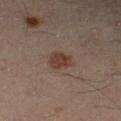Case summary:
• follow-up · catalogued during a skin exam; not biopsied
• imaging modality · 15 mm crop, total-body photography
• location · the left lower leg
• tile lighting · cross-polarized
• subject · male, in their 50s
• lesion size · about 3 mm